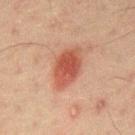Impression:
This lesion was catalogued during total-body skin photography and was not selected for biopsy.
Background:
A male subject aged around 45. Located on the back. A 15 mm close-up extracted from a 3D total-body photography capture.